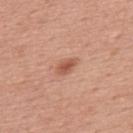Impression:
Captured during whole-body skin photography for melanoma surveillance; the lesion was not biopsied.
Context:
A male subject, about 50 years old. From the upper back. This image is a 15 mm lesion crop taken from a total-body photograph. Captured under white-light illumination. An algorithmic analysis of the crop reported an outline eccentricity of about 0.85 (0 = round, 1 = elongated) and a shape-asymmetry score of about 0.2 (0 = symmetric). And it measured a lesion color around L≈55 a*≈25 b*≈31 in CIELAB, a lesion–skin lightness drop of about 11, and a normalized border contrast of about 7.5.